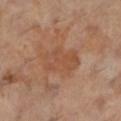{"biopsy_status": "not biopsied; imaged during a skin examination", "site": "left lower leg", "lighting": "cross-polarized", "image": {"source": "total-body photography crop", "field_of_view_mm": 15}, "patient": {"sex": "female", "age_approx": 60}, "lesion_size": {"long_diameter_mm_approx": 5.5}}